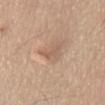Q: Is there a histopathology result?
A: no biopsy performed (imaged during a skin exam)
Q: Lesion size?
A: about 2.5 mm
Q: What is the imaging modality?
A: total-body-photography crop, ~15 mm field of view
Q: Patient demographics?
A: male, aged 58 to 62
Q: What is the anatomic site?
A: the abdomen
Q: Illumination type?
A: white-light